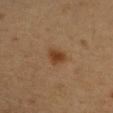Q: What did automated image analysis measure?
A: an average lesion color of about L≈34 a*≈17 b*≈30 (CIELAB) and a lesion-to-skin contrast of about 8.5 (normalized; higher = more distinct)
Q: What are the patient's age and sex?
A: female, in their mid- to late 40s
Q: How was the tile lit?
A: cross-polarized
Q: What is the imaging modality?
A: total-body-photography crop, ~15 mm field of view
Q: Lesion size?
A: about 2.5 mm
Q: Where on the body is the lesion?
A: the right upper arm